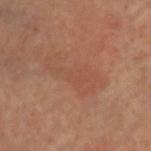workup: imaged on a skin check; not biopsied
anatomic site: the head or neck
patient: male, roughly 55 years of age
lesion size: about 6.5 mm
imaging modality: total-body-photography crop, ~15 mm field of view